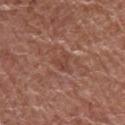biopsy_status: not biopsied; imaged during a skin examination
lesion_size:
  long_diameter_mm_approx: 3.0
image:
  source: total-body photography crop
  field_of_view_mm: 15
automated_metrics:
  border_irregularity_0_10: 4.5
  color_variation_0_10: 2.5
  peripheral_color_asymmetry: 1.0
  nevus_likeness_0_100: 0
  lesion_detection_confidence_0_100: 90
site: right upper arm
patient:
  sex: female
  age_approx: 80